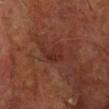follow-up: no biopsy performed (imaged during a skin exam); patient: male, aged around 65; TBP lesion metrics: a lesion color around L≈31 a*≈25 b*≈28 in CIELAB, roughly 6 lightness units darker than nearby skin, and a normalized lesion–skin contrast near 5.5; diameter: about 3 mm; body site: the head or neck; acquisition: total-body-photography crop, ~15 mm field of view.